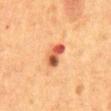No biopsy was performed on this lesion — it was imaged during a full skin examination and was not determined to be concerning.
The tile uses cross-polarized illumination.
Measured at roughly 3 mm in maximum diameter.
The patient is a female aged approximately 50.
A lesion tile, about 15 mm wide, cut from a 3D total-body photograph.
From the abdomen.
The lesion-visualizer software estimated a mean CIELAB color near L≈47 a*≈27 b*≈33, about 15 CIELAB-L* units darker than the surrounding skin, and a normalized border contrast of about 10.5. The analysis additionally found border irregularity of about 3 on a 0–10 scale, a color-variation rating of about 10/10, and radial color variation of about 4.5.